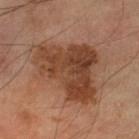<record>
<lighting>cross-polarized</lighting>
<site>left lower leg</site>
<lesion_size>
  <long_diameter_mm_approx>8.5</long_diameter_mm_approx>
</lesion_size>
<patient>
  <sex>male</sex>
  <age_approx>65</age_approx>
</patient>
<image>
  <source>total-body photography crop</source>
  <field_of_view_mm>15</field_of_view_mm>
</image>
</record>